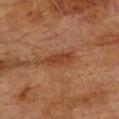biopsy status: catalogued during a skin exam; not biopsied | acquisition: 15 mm crop, total-body photography | diameter: ≈4.5 mm | site: the upper back | patient: male, about 75 years old.